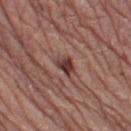notes — imaged on a skin check; not biopsied | illumination — white-light | lesion size — ~2.5 mm (longest diameter) | automated metrics — border irregularity of about 3.5 on a 0–10 scale, internal color variation of about 3 on a 0–10 scale, and peripheral color asymmetry of about 0.5; a nevus-likeness score of about 0/100 and lesion-presence confidence of about 100/100 | patient — male, aged approximately 65 | image source — ~15 mm tile from a whole-body skin photo | body site — the right thigh.